Captured under cross-polarized illumination. A male patient, roughly 50 years of age. This image is a 15 mm lesion crop taken from a total-body photograph. From the back. The lesion's longest dimension is about 2.5 mm — 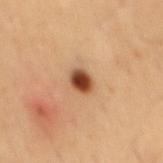On biopsy, histopathology showed an atypical melanocytic neoplasm, classified as an indeterminate (borderline) lesion.